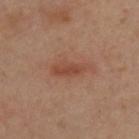notes: no biopsy performed (imaged during a skin exam)
location: the upper back
imaging modality: total-body-photography crop, ~15 mm field of view
lesion size: about 5 mm
subject: male, in their mid- to late 30s
lighting: cross-polarized